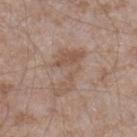– follow-up: imaged on a skin check; not biopsied
– image-analysis metrics: an outline eccentricity of about 0.9 (0 = round, 1 = elongated) and a symmetry-axis asymmetry near 0.65; a border-irregularity index near 9.5/10, internal color variation of about 2.5 on a 0–10 scale, and radial color variation of about 1; an automated nevus-likeness rating near 0 out of 100 and a lesion-detection confidence of about 95/100
– location: the right lower leg
– lesion diameter: ≈6.5 mm
– lighting: white-light illumination
– imaging modality: total-body-photography crop, ~15 mm field of view
– subject: male, aged 43 to 47